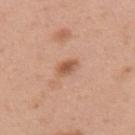Acquisition and patient details: A 15 mm crop from a total-body photograph taken for skin-cancer surveillance. Longest diameter approximately 2.5 mm. The tile uses white-light illumination. The patient is a female aged 48 to 52. Located on the upper back.